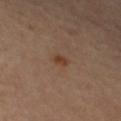Captured during whole-body skin photography for melanoma surveillance; the lesion was not biopsied. A roughly 15 mm field-of-view crop from a total-body skin photograph. The subject is a male aged approximately 65. On the right upper arm.